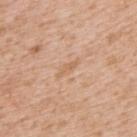* follow-up: total-body-photography surveillance lesion; no biopsy
* anatomic site: the upper back
* lesion size: ~2.5 mm (longest diameter)
* TBP lesion metrics: an area of roughly 2 mm²; a lesion color around L≈63 a*≈20 b*≈35 in CIELAB, roughly 6 lightness units darker than nearby skin, and a lesion-to-skin contrast of about 5 (normalized; higher = more distinct); a classifier nevus-likeness of about 0/100 and lesion-presence confidence of about 100/100
* tile lighting: white-light
* image source: ~15 mm tile from a whole-body skin photo
* patient: male, roughly 65 years of age